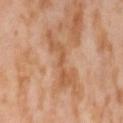{"biopsy_status": "not biopsied; imaged during a skin examination", "image": {"source": "total-body photography crop", "field_of_view_mm": 15}, "lesion_size": {"long_diameter_mm_approx": 6.5}, "patient": {"sex": "female", "age_approx": 55}, "site": "left thigh", "lighting": "cross-polarized"}